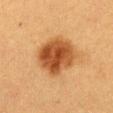Imaged with cross-polarized lighting.
The patient is a female in their 40s.
Longest diameter approximately 5.5 mm.
Automated tile analysis of the lesion measured an area of roughly 19 mm², an eccentricity of roughly 0.5, and two-axis asymmetry of about 0.15. The analysis additionally found a lesion color around L≈41 a*≈22 b*≈35 in CIELAB, about 13 CIELAB-L* units darker than the surrounding skin, and a normalized border contrast of about 10.5. And it measured a border-irregularity rating of about 1.5/10 and a peripheral color-asymmetry measure near 2.
Located on the chest.
A roughly 15 mm field-of-view crop from a total-body skin photograph.